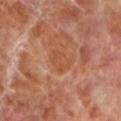workup: imaged on a skin check; not biopsied | automated lesion analysis: a footprint of about 5 mm², an outline eccentricity of about 0.7 (0 = round, 1 = elongated), and two-axis asymmetry of about 0.3; a mean CIELAB color near L≈49 a*≈24 b*≈35; border irregularity of about 3 on a 0–10 scale and a within-lesion color-variation index near 2/10 | imaging modality: 15 mm crop, total-body photography | subject: male, aged 68 to 72 | diameter: ≈3 mm | anatomic site: the left lower leg.